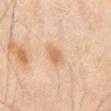{"site": "mid back", "lesion_size": {"long_diameter_mm_approx": 3.0}, "automated_metrics": {"vs_skin_darker_L": 8.0, "vs_skin_contrast_norm": 6.5, "lesion_detection_confidence_0_100": 100}, "patient": {"sex": "male", "age_approx": 70}, "image": {"source": "total-body photography crop", "field_of_view_mm": 15}}